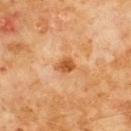Assessment:
Captured during whole-body skin photography for melanoma surveillance; the lesion was not biopsied.
Background:
Located on the chest. Approximately 2.5 mm at its widest. A 15 mm close-up extracted from a 3D total-body photography capture. Automated image analysis of the tile measured a mean CIELAB color near L≈54 a*≈27 b*≈42, about 12 CIELAB-L* units darker than the surrounding skin, and a lesion-to-skin contrast of about 8.5 (normalized; higher = more distinct). It also reported a border-irregularity index near 2.5/10 and a peripheral color-asymmetry measure near 1. It also reported a lesion-detection confidence of about 100/100. This is a cross-polarized tile. A male patient in their 60s.